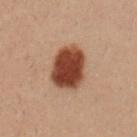Q: Is there a histopathology result?
A: imaged on a skin check; not biopsied
Q: What is the lesion's diameter?
A: ≈4.5 mm
Q: Lesion location?
A: the right upper arm
Q: What lighting was used for the tile?
A: cross-polarized illumination
Q: What is the imaging modality?
A: ~15 mm tile from a whole-body skin photo
Q: What are the patient's age and sex?
A: male, aged 28 to 32